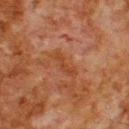This lesion was catalogued during total-body skin photography and was not selected for biopsy.
A male patient roughly 80 years of age.
The lesion's longest dimension is about 4 mm.
Located on the upper back.
An algorithmic analysis of the crop reported a lesion area of about 5 mm², a shape eccentricity near 0.9, and two-axis asymmetry of about 0.35. The software also gave a border-irregularity rating of about 4.5/10, a within-lesion color-variation index near 1/10, and radial color variation of about 0.5. The software also gave a classifier nevus-likeness of about 0/100 and lesion-presence confidence of about 100/100.
A roughly 15 mm field-of-view crop from a total-body skin photograph.